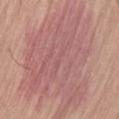Part of a total-body skin-imaging series; this lesion was reviewed on a skin check and was not flagged for biopsy. A roughly 15 mm field-of-view crop from a total-body skin photograph. Located on the lower back. The patient is a male approximately 75 years of age. This is a white-light tile.